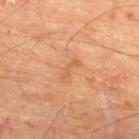notes=imaged on a skin check; not biopsied
patient=male, in their mid- to late 60s
image=15 mm crop, total-body photography
anatomic site=the upper back